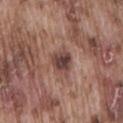The lesion was tiled from a total-body skin photograph and was not biopsied.
Automated tile analysis of the lesion measured a nevus-likeness score of about 10/100 and lesion-presence confidence of about 100/100.
A 15 mm close-up tile from a total-body photography series done for melanoma screening.
Located on the back.
Captured under white-light illumination.
A male patient roughly 75 years of age.